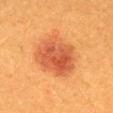Impression: The lesion was photographed on a routine skin check and not biopsied; there is no pathology result. Acquisition and patient details: The lesion is on the mid back. A female patient, aged 38–42. A close-up tile cropped from a whole-body skin photograph, about 15 mm across. Captured under cross-polarized illumination.